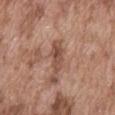No biopsy was performed on this lesion — it was imaged during a full skin examination and was not determined to be concerning. Approximately 4 mm at its widest. The subject is a male in their mid-70s. The tile uses white-light illumination. A roughly 15 mm field-of-view crop from a total-body skin photograph. The lesion is located on the abdomen.